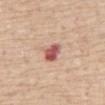{"patient": {"sex": "male", "age_approx": 70}, "lesion_size": {"long_diameter_mm_approx": 3.5}, "lighting": "white-light", "image": {"source": "total-body photography crop", "field_of_view_mm": 15}, "automated_metrics": {"area_mm2_approx": 5.0, "eccentricity": 0.85, "shape_asymmetry": 0.25, "cielab_L": 56, "cielab_a": 29, "cielab_b": 25, "vs_skin_darker_L": 16.0, "vs_skin_contrast_norm": 10.5, "lesion_detection_confidence_0_100": 100}, "site": "abdomen"}